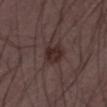Clinical impression: This lesion was catalogued during total-body skin photography and was not selected for biopsy. Image and clinical context: This image is a 15 mm lesion crop taken from a total-body photograph. On the left thigh. A male patient, aged 48 to 52.